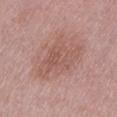<lesion>
<biopsy_status>not biopsied; imaged during a skin examination</biopsy_status>
</lesion>